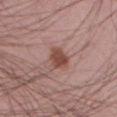notes — catalogued during a skin exam; not biopsied
subject — male, aged around 55
anatomic site — the left thigh
image-analysis metrics — an outline eccentricity of about 0.65 (0 = round, 1 = elongated) and a shape-asymmetry score of about 0.2 (0 = symmetric); an average lesion color of about L≈47 a*≈23 b*≈25 (CIELAB) and a normalized lesion–skin contrast near 9; border irregularity of about 2 on a 0–10 scale, a within-lesion color-variation index near 2/10, and peripheral color asymmetry of about 0.5
imaging modality — ~15 mm tile from a whole-body skin photo
illumination — white-light
lesion size — ≈3 mm